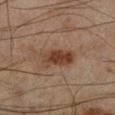Part of a total-body skin-imaging series; this lesion was reviewed on a skin check and was not flagged for biopsy. On the left lower leg. Automated tile analysis of the lesion measured a detector confidence of about 100 out of 100 that the crop contains a lesion. Imaged with cross-polarized lighting. Cropped from a whole-body photographic skin survey; the tile spans about 15 mm. The recorded lesion diameter is about 4.5 mm. The subject is a male in their mid- to late 40s.